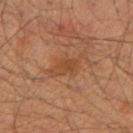{"biopsy_status": "not biopsied; imaged during a skin examination", "lighting": "cross-polarized", "patient": {"sex": "male", "age_approx": 35}, "lesion_size": {"long_diameter_mm_approx": 4.5}, "automated_metrics": {"eccentricity": 0.8, "cielab_L": 42, "cielab_a": 21, "cielab_b": 32, "vs_skin_darker_L": 6.0, "border_irregularity_0_10": 5.0, "color_variation_0_10": 3.0}, "image": {"source": "total-body photography crop", "field_of_view_mm": 15}, "site": "right forearm"}